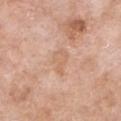From the chest. The tile uses white-light illumination. An algorithmic analysis of the crop reported an area of roughly 5 mm² and a symmetry-axis asymmetry near 0.3. The analysis additionally found border irregularity of about 3.5 on a 0–10 scale and a peripheral color-asymmetry measure near 0.5. The software also gave an automated nevus-likeness rating near 0 out of 100 and a detector confidence of about 100 out of 100 that the crop contains a lesion. A lesion tile, about 15 mm wide, cut from a 3D total-body photograph. A female subject aged approximately 75.